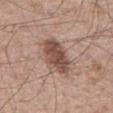notes = catalogued during a skin exam; not biopsied | lesion size = ≈5 mm | subject = male, aged approximately 65 | imaging modality = ~15 mm tile from a whole-body skin photo | automated metrics = an area of roughly 13 mm²; a mean CIELAB color near L≈50 a*≈18 b*≈24, roughly 13 lightness units darker than nearby skin, and a normalized lesion–skin contrast near 9.5 | body site = the abdomen | illumination = white-light illumination.